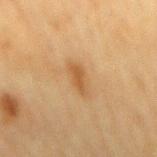| field | value |
|---|---|
| biopsy status | total-body-photography surveillance lesion; no biopsy |
| acquisition | ~15 mm tile from a whole-body skin photo |
| patient | female, roughly 55 years of age |
| location | the mid back |